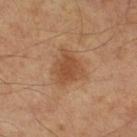Clinical impression:
Captured during whole-body skin photography for melanoma surveillance; the lesion was not biopsied.
Context:
Imaged with cross-polarized lighting. The lesion's longest dimension is about 4.5 mm. A close-up tile cropped from a whole-body skin photograph, about 15 mm across. The patient is a male aged 63 to 67.